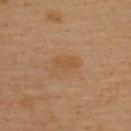notes: no biopsy performed (imaged during a skin exam) | subject: male, approximately 40 years of age | acquisition: 15 mm crop, total-body photography | anatomic site: the upper back | automated lesion analysis: border irregularity of about 2 on a 0–10 scale, internal color variation of about 3 on a 0–10 scale, and radial color variation of about 1; a nevus-likeness score of about 10/100 and lesion-presence confidence of about 100/100 | lesion diameter: ~3.5 mm (longest diameter).